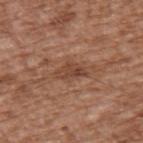Assessment:
Imaged during a routine full-body skin examination; the lesion was not biopsied and no histopathology is available.
Acquisition and patient details:
The tile uses white-light illumination. A close-up tile cropped from a whole-body skin photograph, about 15 mm across. The lesion is on the upper back. A male patient approximately 75 years of age. The total-body-photography lesion software estimated a footprint of about 6.5 mm², an eccentricity of roughly 0.85, and a symmetry-axis asymmetry near 0.3. The software also gave a color-variation rating of about 3.5/10.